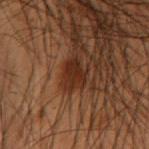biopsy status = total-body-photography surveillance lesion; no biopsy | image = ~15 mm tile from a whole-body skin photo | patient = male, aged 53 to 57 | anatomic site = the right upper arm | automated lesion analysis = an automated nevus-likeness rating near 55 out of 100 | size = ~3.5 mm (longest diameter).